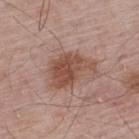biopsy status: catalogued during a skin exam; not biopsied
lighting: white-light
location: the upper back
patient: male, aged around 65
image source: ~15 mm tile from a whole-body skin photo
automated lesion analysis: an eccentricity of roughly 0.8 and two-axis asymmetry of about 0.35; a lesion color around L≈49 a*≈21 b*≈27 in CIELAB, about 11 CIELAB-L* units darker than the surrounding skin, and a lesion-to-skin contrast of about 8.5 (normalized; higher = more distinct); a nevus-likeness score of about 65/100 and a lesion-detection confidence of about 100/100
diameter: ~5.5 mm (longest diameter)